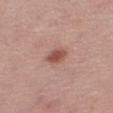Part of a total-body skin-imaging series; this lesion was reviewed on a skin check and was not flagged for biopsy.
Longest diameter approximately 3 mm.
Imaged with white-light lighting.
The lesion is located on the right thigh.
A female patient about 65 years old.
Cropped from a total-body skin-imaging series; the visible field is about 15 mm.